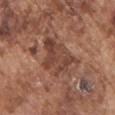{"biopsy_status": "not biopsied; imaged during a skin examination", "patient": {"sex": "male", "age_approx": 75}, "image": {"source": "total-body photography crop", "field_of_view_mm": 15}, "lesion_size": {"long_diameter_mm_approx": 5.0}, "automated_metrics": {"area_mm2_approx": 13.0, "eccentricity": 0.7, "shape_asymmetry": 0.4}, "site": "chest"}